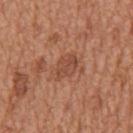{"biopsy_status": "not biopsied; imaged during a skin examination", "site": "mid back", "patient": {"sex": "male", "age_approx": 65}, "image": {"source": "total-body photography crop", "field_of_view_mm": 15}}